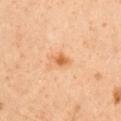Clinical impression: Imaged during a routine full-body skin examination; the lesion was not biopsied and no histopathology is available. Acquisition and patient details: This is a cross-polarized tile. A 15 mm crop from a total-body photograph taken for skin-cancer surveillance. A male subject aged approximately 50. From the chest.